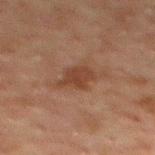No biopsy was performed on this lesion — it was imaged during a full skin examination and was not determined to be concerning.